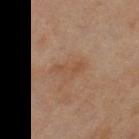| key | value |
|---|---|
| lesion size | ~3.5 mm (longest diameter) |
| subject | female, in their 60s |
| tile lighting | cross-polarized |
| image source | ~15 mm crop, total-body skin-cancer survey |
| body site | the right thigh |
| image-analysis metrics | a lesion area of about 4.5 mm², an outline eccentricity of about 0.9 (0 = round, 1 = elongated), and two-axis asymmetry of about 0.4; a border-irregularity index near 4.5/10 and internal color variation of about 1 on a 0–10 scale; a classifier nevus-likeness of about 0/100 and a detector confidence of about 100 out of 100 that the crop contains a lesion |